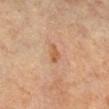Part of a total-body skin-imaging series; this lesion was reviewed on a skin check and was not flagged for biopsy. About 2.5 mm across. A female subject aged around 70. On the right lower leg. Imaged with cross-polarized lighting. A 15 mm close-up tile from a total-body photography series done for melanoma screening.